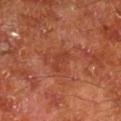anatomic site: the right lower leg | tile lighting: cross-polarized | patient: male, aged 63–67 | image source: ~15 mm crop, total-body skin-cancer survey.